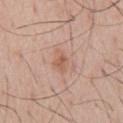Impression:
Part of a total-body skin-imaging series; this lesion was reviewed on a skin check and was not flagged for biopsy.
Context:
This is a white-light tile. A 15 mm close-up tile from a total-body photography series done for melanoma screening. About 3 mm across. The lesion is on the mid back. A male patient, aged 53 to 57.